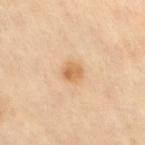The lesion was tiled from a total-body skin photograph and was not biopsied. The lesion's longest dimension is about 2.5 mm. The lesion is located on the right thigh. A roughly 15 mm field-of-view crop from a total-body skin photograph. Captured under cross-polarized illumination. A female subject, aged approximately 70.